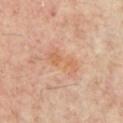patient=aged 53–57; anatomic site=the chest; imaging modality=~15 mm tile from a whole-body skin photo; lesion diameter=about 4.5 mm.